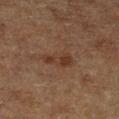workup=catalogued during a skin exam; not biopsied
lesion diameter=~3.5 mm (longest diameter)
lighting=cross-polarized
location=the left lower leg
acquisition=~15 mm crop, total-body skin-cancer survey
subject=male, about 75 years old